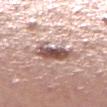notes: catalogued during a skin exam; not biopsied | subject: female, aged approximately 25 | TBP lesion metrics: an automated nevus-likeness rating near 50 out of 100 and lesion-presence confidence of about 100/100 | acquisition: ~15 mm tile from a whole-body skin photo | site: the right lower leg | lesion diameter: ≈5 mm.